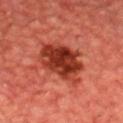Part of a total-body skin-imaging series; this lesion was reviewed on a skin check and was not flagged for biopsy. Imaged with cross-polarized lighting. A close-up tile cropped from a whole-body skin photograph, about 15 mm across. A male subject, approximately 60 years of age. From the head or neck. The total-body-photography lesion software estimated a lesion–skin lightness drop of about 12 and a normalized border contrast of about 11. Approximately 6 mm at its widest.